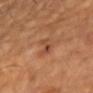Q: Was this lesion biopsied?
A: total-body-photography surveillance lesion; no biopsy
Q: How was the tile lit?
A: cross-polarized illumination
Q: What is the anatomic site?
A: the chest
Q: How was this image acquired?
A: ~15 mm tile from a whole-body skin photo
Q: Who is the patient?
A: male, in their mid-50s